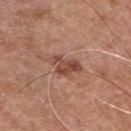Image and clinical context:
The patient is a male in their mid- to late 50s. An algorithmic analysis of the crop reported an average lesion color of about L≈47 a*≈23 b*≈29 (CIELAB) and a lesion-to-skin contrast of about 8 (normalized; higher = more distinct). The software also gave a border-irregularity index near 5/10, a color-variation rating of about 2.5/10, and peripheral color asymmetry of about 0.5. The analysis additionally found a classifier nevus-likeness of about 55/100 and a detector confidence of about 100 out of 100 that the crop contains a lesion. The recorded lesion diameter is about 4 mm. Imaged with white-light lighting. A lesion tile, about 15 mm wide, cut from a 3D total-body photograph. On the chest.